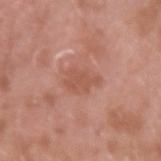Captured during whole-body skin photography for melanoma surveillance; the lesion was not biopsied. Cropped from a total-body skin-imaging series; the visible field is about 15 mm. The lesion's longest dimension is about 3.5 mm. An algorithmic analysis of the crop reported a color-variation rating of about 1.5/10 and radial color variation of about 0.5. The analysis additionally found a nevus-likeness score of about 0/100. The lesion is located on the left forearm. A male subject, aged around 40. The tile uses white-light illumination.